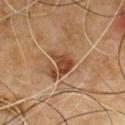Assessment: The lesion was tiled from a total-body skin photograph and was not biopsied. Background: Automated image analysis of the tile measured an area of roughly 5 mm², an outline eccentricity of about 0.7 (0 = round, 1 = elongated), and a symmetry-axis asymmetry near 0.45. It also reported a nevus-likeness score of about 0/100 and lesion-presence confidence of about 95/100. The tile uses cross-polarized illumination. A male patient aged 58 to 62. About 3 mm across. This image is a 15 mm lesion crop taken from a total-body photograph. The lesion is located on the front of the torso.